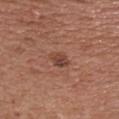The lesion was photographed on a routine skin check and not biopsied; there is no pathology result. Captured under white-light illumination. Located on the chest. Longest diameter approximately 3 mm. A male patient roughly 55 years of age. A close-up tile cropped from a whole-body skin photograph, about 15 mm across.